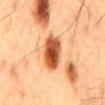A lesion tile, about 15 mm wide, cut from a 3D total-body photograph. Located on the mid back. A male patient, roughly 60 years of age.